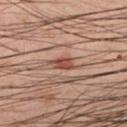Impression: The lesion was photographed on a routine skin check and not biopsied; there is no pathology result. Clinical summary: This is a cross-polarized tile. On the right forearm. A 15 mm crop from a total-body photograph taken for skin-cancer surveillance. The lesion's longest dimension is about 2.5 mm. The patient is a male aged around 40.